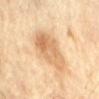The lesion was photographed on a routine skin check and not biopsied; there is no pathology result. A male patient aged 63 to 67. From the lower back. Cropped from a total-body skin-imaging series; the visible field is about 15 mm. The lesion's longest dimension is about 6 mm.